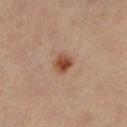No biopsy was performed on this lesion — it was imaged during a full skin examination and was not determined to be concerning. A close-up tile cropped from a whole-body skin photograph, about 15 mm across. The lesion is on the left lower leg. A female patient, aged around 50.